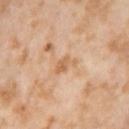Q: Was a biopsy performed?
A: total-body-photography surveillance lesion; no biopsy
Q: Patient demographics?
A: female, in their mid-50s
Q: What kind of image is this?
A: 15 mm crop, total-body photography
Q: What is the anatomic site?
A: the right thigh